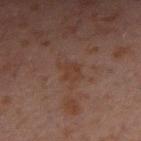Assessment:
This lesion was catalogued during total-body skin photography and was not selected for biopsy.
Image and clinical context:
The lesion is on the right forearm. A lesion tile, about 15 mm wide, cut from a 3D total-body photograph. Automated image analysis of the tile measured a nevus-likeness score of about 0/100. About 2.5 mm across. Imaged with cross-polarized lighting. The subject is a male aged around 30.